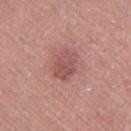Q: Is there a histopathology result?
A: total-body-photography surveillance lesion; no biopsy
Q: Automated lesion metrics?
A: a lesion area of about 7.5 mm², an eccentricity of roughly 0.7, and two-axis asymmetry of about 0.2; an average lesion color of about L≈52 a*≈26 b*≈22 (CIELAB) and about 9 CIELAB-L* units darker than the surrounding skin; border irregularity of about 2 on a 0–10 scale and radial color variation of about 1.5
Q: What lighting was used for the tile?
A: white-light
Q: Lesion size?
A: about 3.5 mm
Q: Lesion location?
A: the leg
Q: How was this image acquired?
A: total-body-photography crop, ~15 mm field of view
Q: What are the patient's age and sex?
A: male, roughly 65 years of age